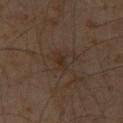Q: Was this lesion biopsied?
A: catalogued during a skin exam; not biopsied
Q: What kind of image is this?
A: ~15 mm crop, total-body skin-cancer survey
Q: Lesion location?
A: the left thigh
Q: Patient demographics?
A: male, about 60 years old